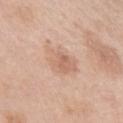Clinical impression:
No biopsy was performed on this lesion — it was imaged during a full skin examination and was not determined to be concerning.
Image and clinical context:
A female subject, aged 38–42. The lesion-visualizer software estimated a lesion color around L≈65 a*≈19 b*≈30 in CIELAB and a normalized border contrast of about 5. It also reported a color-variation rating of about 4/10. It also reported an automated nevus-likeness rating near 0 out of 100 and a detector confidence of about 100 out of 100 that the crop contains a lesion. This image is a 15 mm lesion crop taken from a total-body photograph. The tile uses white-light illumination. About 4.5 mm across. The lesion is located on the left upper arm.